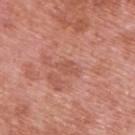size = about 2.5 mm
site = the upper back
lighting = white-light
imaging modality = ~15 mm tile from a whole-body skin photo
subject = male, approximately 70 years of age